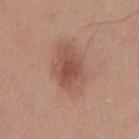Recorded during total-body skin imaging; not selected for excision or biopsy. A 15 mm close-up tile from a total-body photography series done for melanoma screening. The lesion is on the mid back. Imaged with white-light lighting. The patient is a male aged around 30. The recorded lesion diameter is about 4 mm.